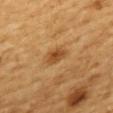Q: Is there a histopathology result?
A: total-body-photography surveillance lesion; no biopsy
Q: How was this image acquired?
A: 15 mm crop, total-body photography
Q: Who is the patient?
A: female, roughly 55 years of age
Q: Lesion location?
A: the upper back
Q: How was the tile lit?
A: cross-polarized
Q: How large is the lesion?
A: about 2.5 mm
Q: Automated lesion metrics?
A: an area of roughly 4 mm² and two-axis asymmetry of about 0.25; a lesion color around L≈49 a*≈23 b*≈42 in CIELAB and a normalized border contrast of about 8; a border-irregularity index near 2.5/10, internal color variation of about 2.5 on a 0–10 scale, and peripheral color asymmetry of about 0.5; lesion-presence confidence of about 100/100